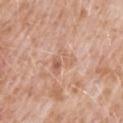workup: no biopsy performed (imaged during a skin exam)
automated lesion analysis: a footprint of about 6.5 mm², an outline eccentricity of about 0.65 (0 = round, 1 = elongated), and a shape-asymmetry score of about 0.35 (0 = symmetric); a mean CIELAB color near L≈62 a*≈21 b*≈31, about 7 CIELAB-L* units darker than the surrounding skin, and a lesion-to-skin contrast of about 4.5 (normalized; higher = more distinct); a border-irregularity index near 4/10, a color-variation rating of about 7/10, and radial color variation of about 3
lesion size: ~3.5 mm (longest diameter)
patient: male, approximately 50 years of age
body site: the mid back
image source: total-body-photography crop, ~15 mm field of view